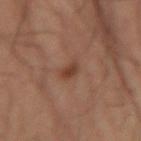follow-up = no biopsy performed (imaged during a skin exam) | patient = male, in their 60s | site = the right forearm | image = total-body-photography crop, ~15 mm field of view | size = ≈2 mm.